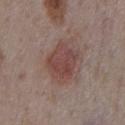image — ~15 mm tile from a whole-body skin photo
automated lesion analysis — roughly 9 lightness units darker than nearby skin and a normalized lesion–skin contrast near 7
lighting — white-light
body site — the chest
lesion size — about 5 mm
subject — male, aged 73–77